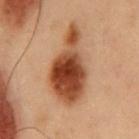Imaged during a routine full-body skin examination; the lesion was not biopsied and no histopathology is available. On the chest. A male subject aged 53 to 57. A 15 mm close-up extracted from a 3D total-body photography capture.